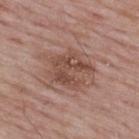This lesion was catalogued during total-body skin photography and was not selected for biopsy. A male subject, aged approximately 65. Cropped from a total-body skin-imaging series; the visible field is about 15 mm. Automated image analysis of the tile measured a mean CIELAB color near L≈48 a*≈20 b*≈26 and a lesion-to-skin contrast of about 7 (normalized; higher = more distinct). The software also gave a border-irregularity index near 5/10 and a within-lesion color-variation index near 4/10. It also reported a detector confidence of about 100 out of 100 that the crop contains a lesion. On the back.